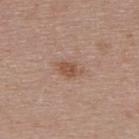Case summary:
– follow-up · catalogued during a skin exam; not biopsied
– illumination · white-light illumination
– automated metrics · an area of roughly 4 mm² and a shape-asymmetry score of about 0.3 (0 = symmetric); border irregularity of about 4 on a 0–10 scale
– lesion size · ≈3 mm
– body site · the upper back
– patient · female, roughly 45 years of age
– imaging modality · total-body-photography crop, ~15 mm field of view